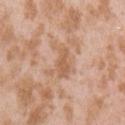Context: A 15 mm close-up extracted from a 3D total-body photography capture. Approximately 4 mm at its widest. The tile uses white-light illumination. The patient is a female aged around 25. Located on the left upper arm.